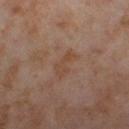{
  "biopsy_status": "not biopsied; imaged during a skin examination",
  "site": "right thigh",
  "lighting": "cross-polarized",
  "lesion_size": {
    "long_diameter_mm_approx": 3.5
  },
  "image": {
    "source": "total-body photography crop",
    "field_of_view_mm": 15
  },
  "patient": {
    "sex": "female",
    "age_approx": 55
  }
}